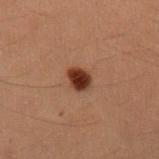Clinical impression:
No biopsy was performed on this lesion — it was imaged during a full skin examination and was not determined to be concerning.
Context:
A lesion tile, about 15 mm wide, cut from a 3D total-body photograph. An algorithmic analysis of the crop reported an average lesion color of about L≈26 a*≈20 b*≈24 (CIELAB) and roughly 14 lightness units darker than nearby skin. Captured under cross-polarized illumination. The recorded lesion diameter is about 2.5 mm. The patient is a female aged around 20. The lesion is located on the left upper arm.